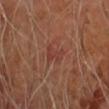<case>
<biopsy_status>not biopsied; imaged during a skin examination</biopsy_status>
<lesion_size>
  <long_diameter_mm_approx>3.0</long_diameter_mm_approx>
</lesion_size>
<image>
  <source>total-body photography crop</source>
  <field_of_view_mm>15</field_of_view_mm>
</image>
<patient>
  <sex>male</sex>
  <age_approx>70</age_approx>
</patient>
<site>right forearm</site>
<automated_metrics>
  <eccentricity>0.8</eccentricity>
  <shape_asymmetry>0.35</shape_asymmetry>
</automated_metrics>
</case>